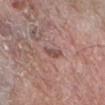Part of a total-body skin-imaging series; this lesion was reviewed on a skin check and was not flagged for biopsy.
Cropped from a total-body skin-imaging series; the visible field is about 15 mm.
A male patient aged 58 to 62.
The recorded lesion diameter is about 2.5 mm.
Located on the right forearm.
The total-body-photography lesion software estimated an automated nevus-likeness rating near 0 out of 100.